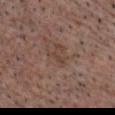TBP lesion metrics = a border-irregularity index near 5.5/10, a within-lesion color-variation index near 0/10, and peripheral color asymmetry of about 0; an automated nevus-likeness rating near 0 out of 100 | lesion size = ~2.5 mm (longest diameter) | anatomic site = the chest | subject = male, aged around 55 | tile lighting = white-light | acquisition = ~15 mm crop, total-body skin-cancer survey.